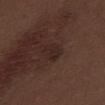biopsy status: imaged on a skin check; not biopsied
imaging modality: ~15 mm crop, total-body skin-cancer survey
patient: male, approximately 70 years of age
lesion diameter: ≈2.5 mm
TBP lesion metrics: an area of roughly 4 mm², an eccentricity of roughly 0.7, and a shape-asymmetry score of about 0.3 (0 = symmetric); peripheral color asymmetry of about 0.5
site: the mid back